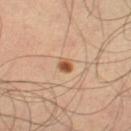  biopsy_status: not biopsied; imaged during a skin examination
  patient:
    sex: male
    age_approx: 65
  site: left thigh
  automated_metrics:
    area_mm2_approx: 2.5
    shape_asymmetry: 0.2
    nevus_likeness_0_100: 100
    lesion_detection_confidence_0_100: 100
  image:
    source: total-body photography crop
    field_of_view_mm: 15
  lesion_size:
    long_diameter_mm_approx: 2.0